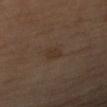Q: Was a biopsy performed?
A: imaged on a skin check; not biopsied
Q: Patient demographics?
A: male, roughly 60 years of age
Q: Lesion location?
A: the arm
Q: What is the imaging modality?
A: ~15 mm crop, total-body skin-cancer survey
Q: What lighting was used for the tile?
A: cross-polarized illumination
Q: How large is the lesion?
A: about 3 mm